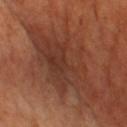{
  "image": {
    "source": "total-body photography crop",
    "field_of_view_mm": 15
  },
  "automated_metrics": {
    "area_mm2_approx": 37.0,
    "shape_asymmetry": 0.3,
    "cielab_L": 35,
    "cielab_a": 22,
    "cielab_b": 27,
    "vs_skin_contrast_norm": 6.5,
    "color_variation_0_10": 4.0,
    "peripheral_color_asymmetry": 1.0
  },
  "lesion_size": {
    "long_diameter_mm_approx": 11.5
  },
  "patient": {
    "sex": "male",
    "age_approx": 65
  },
  "site": "front of the torso",
  "lighting": "cross-polarized"
}